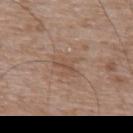No biopsy was performed on this lesion — it was imaged during a full skin examination and was not determined to be concerning.
A male subject, aged approximately 50.
Located on the upper back.
A lesion tile, about 15 mm wide, cut from a 3D total-body photograph.
Longest diameter approximately 3 mm.
This is a white-light tile.